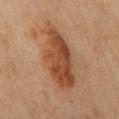Impression: Captured during whole-body skin photography for melanoma surveillance; the lesion was not biopsied. Clinical summary: A female subject aged 43 to 47. The lesion is located on the right upper arm. A close-up tile cropped from a whole-body skin photograph, about 15 mm across. Captured under cross-polarized illumination.